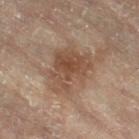| key | value |
|---|---|
| workup | no biopsy performed (imaged during a skin exam) |
| automated metrics | an area of roughly 18 mm², a shape eccentricity near 0.65, and a symmetry-axis asymmetry near 0.3; border irregularity of about 4.5 on a 0–10 scale, a within-lesion color-variation index near 4/10, and a peripheral color-asymmetry measure near 1.5; a classifier nevus-likeness of about 30/100 and a detector confidence of about 100 out of 100 that the crop contains a lesion |
| lesion size | ~6 mm (longest diameter) |
| patient | female, approximately 80 years of age |
| image source | ~15 mm crop, total-body skin-cancer survey |
| illumination | cross-polarized illumination |
| body site | the leg |